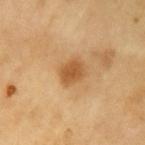Q: Is there a histopathology result?
A: imaged on a skin check; not biopsied
Q: What kind of image is this?
A: total-body-photography crop, ~15 mm field of view
Q: What are the patient's age and sex?
A: male, aged 58–62
Q: Where on the body is the lesion?
A: the left upper arm